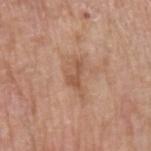Q: Lesion size?
A: ~4 mm (longest diameter)
Q: What are the patient's age and sex?
A: male, approximately 65 years of age
Q: How was the tile lit?
A: white-light
Q: What is the imaging modality?
A: ~15 mm crop, total-body skin-cancer survey
Q: Lesion location?
A: the left upper arm